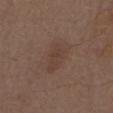Findings:
• biopsy status · no biopsy performed (imaged during a skin exam)
• size · about 4.5 mm
• illumination · white-light
• subject · male, in their 70s
• anatomic site · the mid back
• automated metrics · an area of roughly 9 mm²
• acquisition · ~15 mm tile from a whole-body skin photo